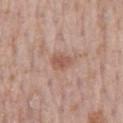  site: lower back
  lighting: white-light
  patient:
    sex: male
    age_approx: 70
  image:
    source: total-body photography crop
    field_of_view_mm: 15
  lesion_size:
    long_diameter_mm_approx: 2.5
  automated_metrics:
    area_mm2_approx: 4.0
    eccentricity: 0.75
    border_irregularity_0_10: 2.5
    color_variation_0_10: 2.0
    peripheral_color_asymmetry: 1.0